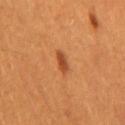Captured during whole-body skin photography for melanoma surveillance; the lesion was not biopsied. The patient is a male approximately 60 years of age. From the back. This is a cross-polarized tile. Measured at roughly 2.5 mm in maximum diameter. A 15 mm close-up tile from a total-body photography series done for melanoma screening.